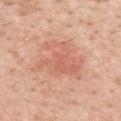notes — imaged on a skin check; not biopsied
location — the upper back
acquisition — ~15 mm crop, total-body skin-cancer survey
lighting — white-light
patient — female, aged around 50